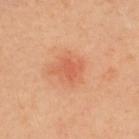workup: imaged on a skin check; not biopsied | site: the upper back | imaging modality: ~15 mm crop, total-body skin-cancer survey | tile lighting: cross-polarized illumination | size: ~3 mm (longest diameter) | patient: female, aged 38 to 42 | automated metrics: an outline eccentricity of about 0.65 (0 = round, 1 = elongated) and a shape-asymmetry score of about 0.25 (0 = symmetric); a lesion–skin lightness drop of about 8 and a normalized border contrast of about 5.5; a border-irregularity rating of about 3.5/10, a color-variation rating of about 2/10, and peripheral color asymmetry of about 1; lesion-presence confidence of about 100/100.